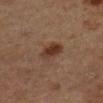Q: Was this lesion biopsied?
A: catalogued during a skin exam; not biopsied
Q: Illumination type?
A: cross-polarized
Q: Who is the patient?
A: male, aged 83 or older
Q: What kind of image is this?
A: ~15 mm crop, total-body skin-cancer survey
Q: Lesion location?
A: the right lower leg
Q: What did automated image analysis measure?
A: a lesion color around L≈30 a*≈17 b*≈24 in CIELAB, a lesion–skin lightness drop of about 8, and a lesion-to-skin contrast of about 9 (normalized; higher = more distinct); a within-lesion color-variation index near 4/10; a nevus-likeness score of about 95/100 and a detector confidence of about 100 out of 100 that the crop contains a lesion